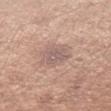The lesion is located on the left forearm. The total-body-photography lesion software estimated a lesion area of about 7.5 mm², an eccentricity of roughly 0.7, and two-axis asymmetry of about 0.2. The software also gave an average lesion color of about L≈58 a*≈17 b*≈22 (CIELAB), a lesion–skin lightness drop of about 8, and a normalized lesion–skin contrast near 6. The recorded lesion diameter is about 3.5 mm. The patient is a female in their 30s. A roughly 15 mm field-of-view crop from a total-body skin photograph. Imaged with white-light lighting.